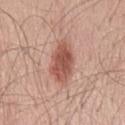| field | value |
|---|---|
| notes | catalogued during a skin exam; not biopsied |
| site | the mid back |
| automated metrics | an area of roughly 12 mm² and an outline eccentricity of about 0.85 (0 = round, 1 = elongated); about 13 CIELAB-L* units darker than the surrounding skin and a normalized lesion–skin contrast near 9; a nevus-likeness score of about 90/100 |
| image source | total-body-photography crop, ~15 mm field of view |
| patient | male, in their mid- to late 60s |
| lighting | white-light |
| lesion diameter | about 5.5 mm |